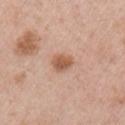biopsy status — total-body-photography surveillance lesion; no biopsy | site — the left upper arm | size — ≈3 mm | imaging modality — ~15 mm crop, total-body skin-cancer survey | patient — female, aged approximately 50 | lighting — white-light illumination.